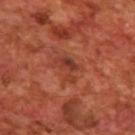Q: Is there a histopathology result?
A: catalogued during a skin exam; not biopsied
Q: Patient demographics?
A: male, aged 68–72
Q: What is the imaging modality?
A: total-body-photography crop, ~15 mm field of view
Q: How was the tile lit?
A: cross-polarized illumination
Q: What is the anatomic site?
A: the back
Q: What is the lesion's diameter?
A: ≈3.5 mm